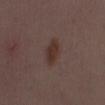Imaged during a routine full-body skin examination; the lesion was not biopsied and no histopathology is available. Longest diameter approximately 4 mm. This is a white-light tile. A female patient, aged 28 to 32. Located on the front of the torso. A 15 mm close-up extracted from a 3D total-body photography capture.